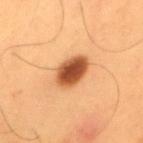notes — no biopsy performed (imaged during a skin exam)
diameter — ~4.5 mm (longest diameter)
site — the upper back
image source — 15 mm crop, total-body photography
lighting — cross-polarized
subject — male, aged 53–57
image-analysis metrics — a footprint of about 10 mm², an eccentricity of roughly 0.8, and a symmetry-axis asymmetry near 0.1; an average lesion color of about L≈53 a*≈28 b*≈40 (CIELAB), about 20 CIELAB-L* units darker than the surrounding skin, and a normalized lesion–skin contrast near 12.5; a border-irregularity index near 1.5/10 and a color-variation rating of about 6/10; a classifier nevus-likeness of about 100/100